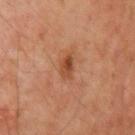Acquisition and patient details:
A male patient about 60 years old. From the mid back. The lesion-visualizer software estimated a footprint of about 4.5 mm², an outline eccentricity of about 0.85 (0 = round, 1 = elongated), and a shape-asymmetry score of about 0.25 (0 = symmetric). The software also gave a classifier nevus-likeness of about 30/100 and a lesion-detection confidence of about 100/100. A lesion tile, about 15 mm wide, cut from a 3D total-body photograph. The recorded lesion diameter is about 3 mm. The tile uses cross-polarized illumination.